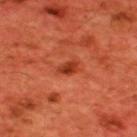Q: What is the anatomic site?
A: the upper back
Q: What kind of image is this?
A: ~15 mm crop, total-body skin-cancer survey
Q: How large is the lesion?
A: ≈2.5 mm
Q: What are the patient's age and sex?
A: male, about 60 years old